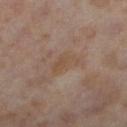biopsy_status: not biopsied; imaged during a skin examination
site: left lower leg
lighting: cross-polarized
patient:
  sex: female
  age_approx: 55
lesion_size:
  long_diameter_mm_approx: 3.0
image:
  source: total-body photography crop
  field_of_view_mm: 15